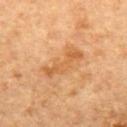Findings:
– workup — imaged on a skin check; not biopsied
– patient — female, aged approximately 60
– lighting — cross-polarized illumination
– size — ≈5.5 mm
– location — the upper back
– imaging modality — total-body-photography crop, ~15 mm field of view